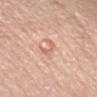Clinical impression: The lesion was tiled from a total-body skin photograph and was not biopsied. Image and clinical context: An algorithmic analysis of the crop reported a footprint of about 3.5 mm². It also reported a color-variation rating of about 0/10. The software also gave an automated nevus-likeness rating near 0 out of 100 and a detector confidence of about 100 out of 100 that the crop contains a lesion. Measured at roughly 2.5 mm in maximum diameter. The patient is a female aged 63 to 67. A region of skin cropped from a whole-body photographic capture, roughly 15 mm wide. Located on the head or neck. This is a white-light tile.